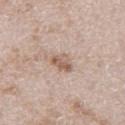{
  "biopsy_status": "not biopsied; imaged during a skin examination",
  "lesion_size": {
    "long_diameter_mm_approx": 2.5
  },
  "site": "leg",
  "lighting": "white-light",
  "image": {
    "source": "total-body photography crop",
    "field_of_view_mm": 15
  },
  "patient": {
    "sex": "male",
    "age_approx": 65
  }
}